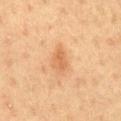Assessment:
Imaged during a routine full-body skin examination; the lesion was not biopsied and no histopathology is available.